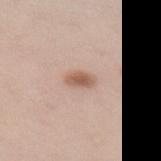Impression:
No biopsy was performed on this lesion — it was imaged during a full skin examination and was not determined to be concerning.